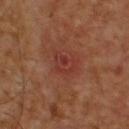anatomic site — the upper back
image source — ~15 mm tile from a whole-body skin photo
lighting — cross-polarized illumination
lesion size — ~3.5 mm (longest diameter)
patient — male, aged 58 to 62